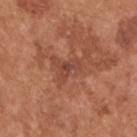workup: catalogued during a skin exam; not biopsied | patient: male, in their mid- to late 50s | image source: ~15 mm crop, total-body skin-cancer survey | lighting: white-light | size: ≈3 mm | automated lesion analysis: a footprint of about 4.5 mm², an eccentricity of roughly 0.8, and a shape-asymmetry score of about 0.6 (0 = symmetric); about 7 CIELAB-L* units darker than the surrounding skin and a normalized lesion–skin contrast near 6 | body site: the upper back.